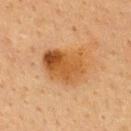The lesion is on the back. The lesion-visualizer software estimated a lesion area of about 17 mm² and two-axis asymmetry of about 0.2. The analysis additionally found a normalized border contrast of about 9. The analysis additionally found a border-irregularity index near 2/10, a color-variation rating of about 8/10, and a peripheral color-asymmetry measure near 3. It also reported a nevus-likeness score of about 70/100 and a detector confidence of about 100 out of 100 that the crop contains a lesion. The patient is a female aged 38 to 42. Measured at roughly 5 mm in maximum diameter. A 15 mm crop from a total-body photograph taken for skin-cancer surveillance.